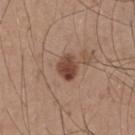Q: Was a biopsy performed?
A: total-body-photography surveillance lesion; no biopsy
Q: Lesion size?
A: ≈3 mm
Q: What lighting was used for the tile?
A: white-light illumination
Q: What did automated image analysis measure?
A: a border-irregularity index near 1.5/10
Q: How was this image acquired?
A: total-body-photography crop, ~15 mm field of view
Q: Lesion location?
A: the upper back
Q: What are the patient's age and sex?
A: male, approximately 50 years of age